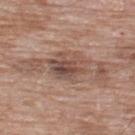follow-up — catalogued during a skin exam; not biopsied
image — ~15 mm tile from a whole-body skin photo
subject — male, about 60 years old
body site — the back
diameter — ~9.5 mm (longest diameter)
tile lighting — white-light